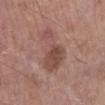The lesion was photographed on a routine skin check and not biopsied; there is no pathology result. The recorded lesion diameter is about 6.5 mm. A male patient, aged 58–62. The total-body-photography lesion software estimated a footprint of about 14 mm², a shape eccentricity near 0.9, and a symmetry-axis asymmetry near 0.5. And it measured an average lesion color of about L≈48 a*≈21 b*≈23 (CIELAB) and a normalized lesion–skin contrast near 6.5. The analysis additionally found a border-irregularity index near 6/10, a within-lesion color-variation index near 3.5/10, and radial color variation of about 1. The software also gave a nevus-likeness score of about 45/100. Located on the left lower leg. This is a white-light tile. A region of skin cropped from a whole-body photographic capture, roughly 15 mm wide.